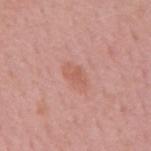Case summary:
* patient: male, aged approximately 60
* TBP lesion metrics: an average lesion color of about L≈58 a*≈26 b*≈28 (CIELAB) and a normalized lesion–skin contrast near 5.5; internal color variation of about 1.5 on a 0–10 scale and a peripheral color-asymmetry measure near 0.5; a detector confidence of about 100 out of 100 that the crop contains a lesion
* site: the mid back
* image: total-body-photography crop, ~15 mm field of view
* diameter: about 3 mm